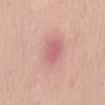Q: Was this lesion biopsied?
A: no biopsy performed (imaged during a skin exam)
Q: How large is the lesion?
A: ≈5 mm
Q: How was the tile lit?
A: white-light
Q: How was this image acquired?
A: total-body-photography crop, ~15 mm field of view
Q: Who is the patient?
A: male, aged approximately 50
Q: Lesion location?
A: the abdomen